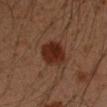No biopsy was performed on this lesion — it was imaged during a full skin examination and was not determined to be concerning. A 15 mm close-up extracted from a 3D total-body photography capture. The subject is a male roughly 50 years of age. The lesion is on the left upper arm.